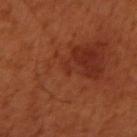Impression:
Imaged during a routine full-body skin examination; the lesion was not biopsied and no histopathology is available.
Background:
On the right upper arm. Approximately 1.5 mm at its widest. A male patient, aged approximately 50. A 15 mm close-up extracted from a 3D total-body photography capture. This is a cross-polarized tile.